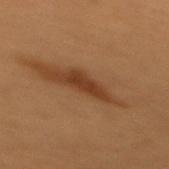<lesion>
  <biopsy_status>not biopsied; imaged during a skin examination</biopsy_status>
  <lesion_size>
    <long_diameter_mm_approx>4.5</long_diameter_mm_approx>
  </lesion_size>
  <image>
    <source>total-body photography crop</source>
    <field_of_view_mm>15</field_of_view_mm>
  </image>
  <patient>
    <sex>male</sex>
    <age_approx>50</age_approx>
  </patient>
  <site>mid back</site>
</lesion>